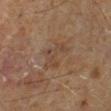Imaged during a routine full-body skin examination; the lesion was not biopsied and no histopathology is available. From the left lower leg. The tile uses cross-polarized illumination. A 15 mm close-up tile from a total-body photography series done for melanoma screening. The patient is a male about 60 years old. Measured at roughly 4.5 mm in maximum diameter. Automated tile analysis of the lesion measured an average lesion color of about L≈41 a*≈15 b*≈27 (CIELAB) and a normalized lesion–skin contrast near 4.5. The software also gave a nevus-likeness score of about 0/100 and a lesion-detection confidence of about 100/100.